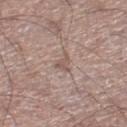Impression:
The lesion was tiled from a total-body skin photograph and was not biopsied.
Acquisition and patient details:
From the right lower leg. A male patient, aged around 60. Imaged with white-light lighting. The recorded lesion diameter is about 2.5 mm. Automated tile analysis of the lesion measured a lesion area of about 3 mm², a shape eccentricity near 0.65, and a shape-asymmetry score of about 0.6 (0 = symmetric). And it measured border irregularity of about 6 on a 0–10 scale, internal color variation of about 0.5 on a 0–10 scale, and a peripheral color-asymmetry measure near 0. A lesion tile, about 15 mm wide, cut from a 3D total-body photograph.